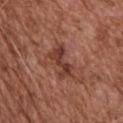Q: Was a biopsy performed?
A: no biopsy performed (imaged during a skin exam)
Q: Patient demographics?
A: male, in their mid- to late 70s
Q: What is the imaging modality?
A: total-body-photography crop, ~15 mm field of view
Q: Illumination type?
A: white-light illumination
Q: Lesion location?
A: the upper back
Q: How large is the lesion?
A: ≈4 mm